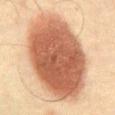Recorded during total-body skin imaging; not selected for excision or biopsy. Imaged with cross-polarized lighting. The lesion is on the abdomen. The subject is a male about 60 years old. Automated image analysis of the tile measured an average lesion color of about L≈49 a*≈21 b*≈29 (CIELAB) and a normalized lesion–skin contrast near 12. It also reported a within-lesion color-variation index near 5.5/10 and radial color variation of about 1.5. And it measured a classifier nevus-likeness of about 100/100 and lesion-presence confidence of about 100/100. Approximately 12 mm at its widest. A region of skin cropped from a whole-body photographic capture, roughly 15 mm wide.